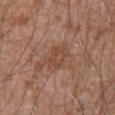Q: Is there a histopathology result?
A: no biopsy performed (imaged during a skin exam)
Q: How was this image acquired?
A: ~15 mm tile from a whole-body skin photo
Q: Lesion size?
A: ≈2.5 mm
Q: What is the anatomic site?
A: the mid back
Q: Who is the patient?
A: male, aged approximately 60
Q: Automated lesion metrics?
A: a footprint of about 4 mm² and a shape eccentricity near 0.7; a color-variation rating of about 3/10; a classifier nevus-likeness of about 0/100